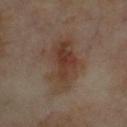Q: Was a biopsy performed?
A: catalogued during a skin exam; not biopsied
Q: What is the anatomic site?
A: the front of the torso
Q: What kind of image is this?
A: 15 mm crop, total-body photography
Q: How large is the lesion?
A: about 6.5 mm
Q: Illumination type?
A: cross-polarized illumination
Q: Who is the patient?
A: male, aged 63–67